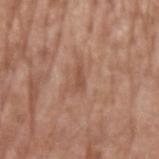The lesion was photographed on a routine skin check and not biopsied; there is no pathology result. A male subject, in their mid-70s. Located on the arm. Cropped from a whole-body photographic skin survey; the tile spans about 15 mm.